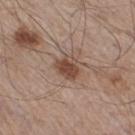| field | value |
|---|---|
| workup | no biopsy performed (imaged during a skin exam) |
| location | the right thigh |
| image source | 15 mm crop, total-body photography |
| subject | male, about 60 years old |